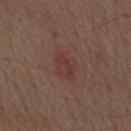Findings:
* follow-up: imaged on a skin check; not biopsied
* subject: male, in their 70s
* illumination: white-light illumination
* imaging modality: total-body-photography crop, ~15 mm field of view
* anatomic site: the mid back